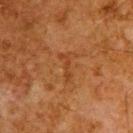Q: Is there a histopathology result?
A: catalogued during a skin exam; not biopsied
Q: Who is the patient?
A: male, aged around 80
Q: How was the tile lit?
A: cross-polarized illumination
Q: What kind of image is this?
A: ~15 mm tile from a whole-body skin photo
Q: How large is the lesion?
A: ~3.5 mm (longest diameter)
Q: Lesion location?
A: the upper back